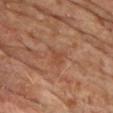| field | value |
|---|---|
| biopsy status | imaged on a skin check; not biopsied |
| patient | male, in their mid- to late 60s |
| size | about 2.5 mm |
| automated metrics | an area of roughly 3.5 mm², a shape eccentricity near 0.6, and a symmetry-axis asymmetry near 0.4; a lesion color around L≈45 a*≈23 b*≈31 in CIELAB and a lesion–skin lightness drop of about 5 |
| site | the front of the torso |
| image | total-body-photography crop, ~15 mm field of view |